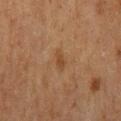Captured during whole-body skin photography for melanoma surveillance; the lesion was not biopsied.
From the mid back.
A male patient, aged 73 to 77.
A close-up tile cropped from a whole-body skin photograph, about 15 mm across.
Automated image analysis of the tile measured a footprint of about 2.5 mm² and two-axis asymmetry of about 0.3. The software also gave roughly 6 lightness units darker than nearby skin and a normalized border contrast of about 6. The software also gave a border-irregularity index near 3.5/10, internal color variation of about 0 on a 0–10 scale, and peripheral color asymmetry of about 0.
The tile uses cross-polarized illumination.